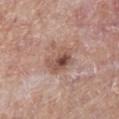No biopsy was performed on this lesion — it was imaged during a full skin examination and was not determined to be concerning.
From the right lower leg.
A female patient, aged around 70.
A roughly 15 mm field-of-view crop from a total-body skin photograph.